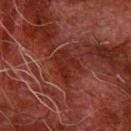| key | value |
|---|---|
| biopsy status | no biopsy performed (imaged during a skin exam) |
| body site | the right forearm |
| image source | ~15 mm tile from a whole-body skin photo |
| lesion size | ≈4 mm |
| tile lighting | cross-polarized illumination |
| patient | male, aged approximately 80 |